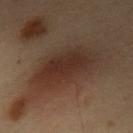Recorded during total-body skin imaging; not selected for excision or biopsy.
A roughly 15 mm field-of-view crop from a total-body skin photograph.
Imaged with cross-polarized lighting.
Automated tile analysis of the lesion measured an average lesion color of about L≈24 a*≈14 b*≈19 (CIELAB), a lesion–skin lightness drop of about 7, and a normalized border contrast of about 8. And it measured a nevus-likeness score of about 0/100 and lesion-presence confidence of about 100/100.
On the chest.
The subject is a male aged 53–57.
The recorded lesion diameter is about 7 mm.